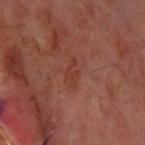The lesion was photographed on a routine skin check and not biopsied; there is no pathology result. The lesion-visualizer software estimated an area of roughly 3 mm² and a symmetry-axis asymmetry near 0.4. It also reported a mean CIELAB color near L≈36 a*≈25 b*≈28, about 5 CIELAB-L* units darker than the surrounding skin, and a lesion-to-skin contrast of about 5 (normalized; higher = more distinct). The software also gave a border-irregularity index near 5.5/10 and peripheral color asymmetry of about 0. The software also gave a lesion-detection confidence of about 100/100. From the left upper arm. A male patient, in their mid- to late 60s. A roughly 15 mm field-of-view crop from a total-body skin photograph. Captured under cross-polarized illumination. Approximately 3 mm at its widest.